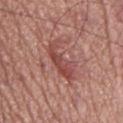Impression: Recorded during total-body skin imaging; not selected for excision or biopsy. Context: The lesion's longest dimension is about 6 mm. Imaged with white-light lighting. A 15 mm close-up tile from a total-body photography series done for melanoma screening. A male patient aged approximately 75. The lesion-visualizer software estimated a border-irregularity rating of about 6/10 and a color-variation rating of about 3.5/10. The software also gave a nevus-likeness score of about 5/100 and a detector confidence of about 90 out of 100 that the crop contains a lesion. The lesion is located on the mid back.